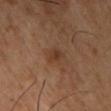Case summary:
– workup: no biopsy performed (imaged during a skin exam)
– site: the chest
– patient: male, aged 53 to 57
– imaging modality: 15 mm crop, total-body photography